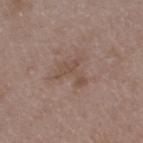Assessment:
No biopsy was performed on this lesion — it was imaged during a full skin examination and was not determined to be concerning.
Clinical summary:
A 15 mm close-up extracted from a 3D total-body photography capture. The lesion is located on the left thigh. A male patient, aged approximately 50.